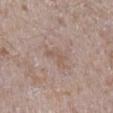Part of a total-body skin-imaging series; this lesion was reviewed on a skin check and was not flagged for biopsy. The lesion's longest dimension is about 3 mm. The lesion is on the right lower leg. A male patient, aged around 45. A roughly 15 mm field-of-view crop from a total-body skin photograph.